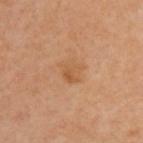{
  "biopsy_status": "not biopsied; imaged during a skin examination",
  "lesion_size": {
    "long_diameter_mm_approx": 3.0
  },
  "site": "upper back",
  "automated_metrics": {
    "cielab_L": 55,
    "cielab_a": 21,
    "cielab_b": 37,
    "vs_skin_darker_L": 6.0,
    "vs_skin_contrast_norm": 5.5
  },
  "image": {
    "source": "total-body photography crop",
    "field_of_view_mm": 15
  },
  "patient": {
    "sex": "male",
    "age_approx": 40
  },
  "lighting": "cross-polarized"
}